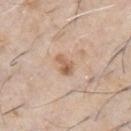{"biopsy_status": "not biopsied; imaged during a skin examination", "image": {"source": "total-body photography crop", "field_of_view_mm": 15}, "site": "chest", "lesion_size": {"long_diameter_mm_approx": 3.0}, "patient": {"sex": "male", "age_approx": 60}, "lighting": "white-light"}